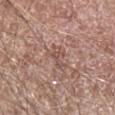  biopsy_status: not biopsied; imaged during a skin examination
  lighting: white-light
  image:
    source: total-body photography crop
    field_of_view_mm: 15
  automated_metrics:
    area_mm2_approx: 3.0
    eccentricity: 0.9
    cielab_L: 51
    cielab_a: 19
    cielab_b: 24
    vs_skin_darker_L: 7.0
    border_irregularity_0_10: 5.5
    color_variation_0_10: 0.0
    peripheral_color_asymmetry: 0.0
  patient:
    sex: male
    age_approx: 60
  site: arm
  lesion_size:
    long_diameter_mm_approx: 3.0